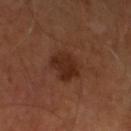No biopsy was performed on this lesion — it was imaged during a full skin examination and was not determined to be concerning. The patient is a male aged approximately 65. A close-up tile cropped from a whole-body skin photograph, about 15 mm across.